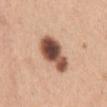Notes:
* notes · total-body-photography surveillance lesion; no biopsy
* body site · the chest
* imaging modality · 15 mm crop, total-body photography
* subject · female, approximately 30 years of age
* image-analysis metrics · an area of roughly 14 mm², a shape eccentricity near 0.85, and two-axis asymmetry of about 0.25
* lesion size · ~5.5 mm (longest diameter)
* tile lighting · white-light